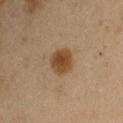| field | value |
|---|---|
| biopsy status | imaged on a skin check; not biopsied |
| automated lesion analysis | a normalized lesion–skin contrast near 9.5; an automated nevus-likeness rating near 100 out of 100 and a detector confidence of about 100 out of 100 that the crop contains a lesion |
| anatomic site | the right upper arm |
| acquisition | ~15 mm tile from a whole-body skin photo |
| lesion diameter | about 4 mm |
| subject | female, approximately 40 years of age |
| tile lighting | cross-polarized |